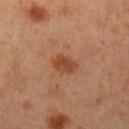Imaged during a routine full-body skin examination; the lesion was not biopsied and no histopathology is available. This image is a 15 mm lesion crop taken from a total-body photograph. The recorded lesion diameter is about 3.5 mm. On the left upper arm. The subject is a female roughly 40 years of age. The tile uses cross-polarized illumination.